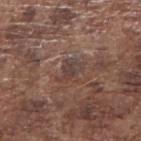Assessment: The lesion was photographed on a routine skin check and not biopsied; there is no pathology result. Background: A male subject, aged approximately 75. A close-up tile cropped from a whole-body skin photograph, about 15 mm across. Measured at roughly 3 mm in maximum diameter. On the right upper arm. The total-body-photography lesion software estimated lesion-presence confidence of about 65/100.